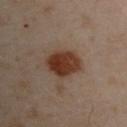Part of a total-body skin-imaging series; this lesion was reviewed on a skin check and was not flagged for biopsy.
A male patient aged approximately 50.
Automated tile analysis of the lesion measured a mean CIELAB color near L≈27 a*≈17 b*≈23 and a lesion–skin lightness drop of about 11. And it measured a border-irregularity index near 1.5/10, internal color variation of about 4 on a 0–10 scale, and a peripheral color-asymmetry measure near 1.5.
The lesion is located on the left arm.
A 15 mm close-up tile from a total-body photography series done for melanoma screening.
This is a cross-polarized tile.